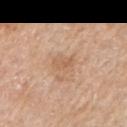The lesion was tiled from a total-body skin photograph and was not biopsied.
The lesion is located on the left upper arm.
Cropped from a total-body skin-imaging series; the visible field is about 15 mm.
A male patient, roughly 60 years of age.
This is a white-light tile.
The lesion's longest dimension is about 2.5 mm.